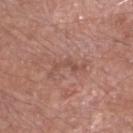Captured during whole-body skin photography for melanoma surveillance; the lesion was not biopsied. A close-up tile cropped from a whole-body skin photograph, about 15 mm across. Automated image analysis of the tile measured an outline eccentricity of about 0.95 (0 = round, 1 = elongated) and two-axis asymmetry of about 0.65. The patient is a male aged 58–62. Located on the head or neck. Captured under white-light illumination. The lesion's longest dimension is about 4.5 mm.